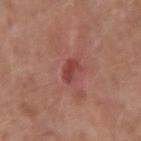Clinical impression:
No biopsy was performed on this lesion — it was imaged during a full skin examination and was not determined to be concerning.
Background:
A 15 mm close-up tile from a total-body photography series done for melanoma screening. From the left upper arm. A female subject, roughly 60 years of age. Automated tile analysis of the lesion measured a shape eccentricity near 0.8. It also reported an average lesion color of about L≈43 a*≈29 b*≈25 (CIELAB), roughly 9 lightness units darker than nearby skin, and a lesion-to-skin contrast of about 7 (normalized; higher = more distinct).